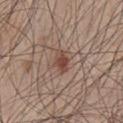Assessment:
The lesion was photographed on a routine skin check and not biopsied; there is no pathology result.
Clinical summary:
Cropped from a whole-body photographic skin survey; the tile spans about 15 mm. Automated image analysis of the tile measured an area of roughly 4.5 mm², an outline eccentricity of about 0.75 (0 = round, 1 = elongated), and two-axis asymmetry of about 0.2. The software also gave a lesion color around L≈46 a*≈19 b*≈25 in CIELAB and a normalized lesion–skin contrast near 8. And it measured a classifier nevus-likeness of about 95/100 and a lesion-detection confidence of about 100/100. A male subject in their 50s. Imaged with white-light lighting. From the chest. Approximately 3 mm at its widest.